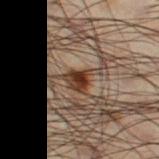Q: Is there a histopathology result?
A: total-body-photography surveillance lesion; no biopsy
Q: Illumination type?
A: cross-polarized
Q: What is the imaging modality?
A: 15 mm crop, total-body photography
Q: Lesion size?
A: ~4 mm (longest diameter)
Q: Lesion location?
A: the right thigh
Q: Who is the patient?
A: male, roughly 50 years of age